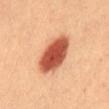Clinical impression:
Recorded during total-body skin imaging; not selected for excision or biopsy.
Acquisition and patient details:
A female patient in their 30s. About 5.5 mm across. A close-up tile cropped from a whole-body skin photograph, about 15 mm across. From the abdomen. The total-body-photography lesion software estimated a lesion color around L≈56 a*≈33 b*≈37 in CIELAB, roughly 21 lightness units darker than nearby skin, and a normalized lesion–skin contrast near 12.5. And it measured peripheral color asymmetry of about 1.5. Imaged with cross-polarized lighting.